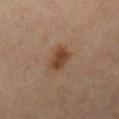notes — imaged on a skin check; not biopsied | tile lighting — cross-polarized | anatomic site — the right lower leg | size — ~3 mm (longest diameter) | automated lesion analysis — a lesion area of about 6 mm² and a shape eccentricity near 0.6; a lesion color around L≈36 a*≈17 b*≈27 in CIELAB, roughly 9 lightness units darker than nearby skin, and a lesion-to-skin contrast of about 9 (normalized; higher = more distinct); a nevus-likeness score of about 95/100 and a detector confidence of about 100 out of 100 that the crop contains a lesion | image — ~15 mm tile from a whole-body skin photo | patient — female, aged 68–72.